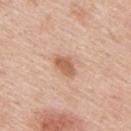| feature | finding |
|---|---|
| notes | no biopsy performed (imaged during a skin exam) |
| body site | the right upper arm |
| patient | male, about 40 years old |
| lighting | white-light |
| imaging modality | total-body-photography crop, ~15 mm field of view |
| diameter | ~3 mm (longest diameter) |